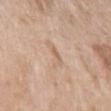Impression: The lesion was tiled from a total-body skin photograph and was not biopsied. Context: The patient is a male in their 60s. On the left upper arm. Automated tile analysis of the lesion measured an area of roughly 4.5 mm², a shape eccentricity near 0.85, and a shape-asymmetry score of about 0.5 (0 = symmetric). It also reported an average lesion color of about L≈64 a*≈16 b*≈31 (CIELAB), a lesion–skin lightness drop of about 6, and a lesion-to-skin contrast of about 4.5 (normalized; higher = more distinct). And it measured a color-variation rating of about 2.5/10. Approximately 3.5 mm at its widest. Cropped from a whole-body photographic skin survey; the tile spans about 15 mm.